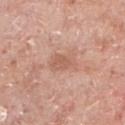{"site": "left lower leg", "image": {"source": "total-body photography crop", "field_of_view_mm": 15}, "patient": {"sex": "male", "age_approx": 65}}